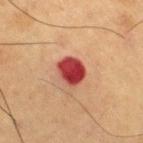Captured during whole-body skin photography for melanoma surveillance; the lesion was not biopsied. From the leg. This image is a 15 mm lesion crop taken from a total-body photograph. The subject is a male aged approximately 55.